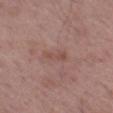site: left thigh
lighting: white-light
lesion_size:
  long_diameter_mm_approx: 3.0
patient:
  sex: male
  age_approx: 50
image:
  source: total-body photography crop
  field_of_view_mm: 15